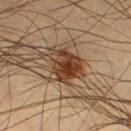{
  "biopsy_status": "not biopsied; imaged during a skin examination",
  "lesion_size": {
    "long_diameter_mm_approx": 4.5
  },
  "image": {
    "source": "total-body photography crop",
    "field_of_view_mm": 15
  },
  "site": "leg",
  "patient": {
    "sex": "male",
    "age_approx": 35
  }
}